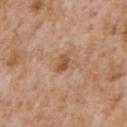The patient is a male aged around 65. A roughly 15 mm field-of-view crop from a total-body skin photograph. Captured under white-light illumination. On the front of the torso.